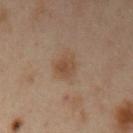A 15 mm close-up tile from a total-body photography series done for melanoma screening. The subject is a female aged approximately 40. The lesion is located on the left upper arm.